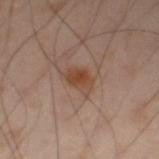- follow-up · no biopsy performed (imaged during a skin exam)
- patient · male, aged approximately 55
- location · the leg
- lighting · cross-polarized illumination
- image source · total-body-photography crop, ~15 mm field of view